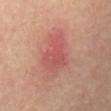Assessment: Recorded during total-body skin imaging; not selected for excision or biopsy. Image and clinical context: The patient is a male aged 68–72. Cropped from a total-body skin-imaging series; the visible field is about 15 mm. The lesion's longest dimension is about 4.5 mm. From the abdomen.